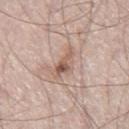No biopsy was performed on this lesion — it was imaged during a full skin examination and was not determined to be concerning. A 15 mm close-up extracted from a 3D total-body photography capture. A male subject in their mid- to late 50s. The tile uses white-light illumination. From the right thigh.